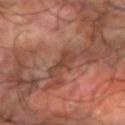<case>
<biopsy_status>not biopsied; imaged during a skin examination</biopsy_status>
<site>arm</site>
<lighting>cross-polarized</lighting>
<lesion_size>
  <long_diameter_mm_approx>3.0</long_diameter_mm_approx>
</lesion_size>
<image>
  <source>total-body photography crop</source>
  <field_of_view_mm>15</field_of_view_mm>
</image>
<automated_metrics>
  <nevus_likeness_0_100>0</nevus_likeness_0_100>
</automated_metrics>
<patient>
  <sex>male</sex>
  <age_approx>60</age_approx>
</patient>
</case>